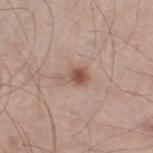biopsy_status: not biopsied; imaged during a skin examination
site: left lower leg
patient:
  sex: male
  age_approx: 60
lighting: white-light
automated_metrics:
  area_mm2_approx: 4.5
  eccentricity: 0.75
  shape_asymmetry: 0.45
  cielab_L: 53
  cielab_a: 20
  cielab_b: 26
  vs_skin_darker_L: 11.0
image:
  source: total-body photography crop
  field_of_view_mm: 15